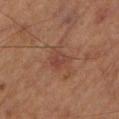biopsy status: total-body-photography surveillance lesion; no biopsy
subject: male, aged around 85 or older
anatomic site: the left lower leg
acquisition: total-body-photography crop, ~15 mm field of view